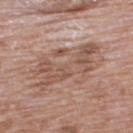| feature | finding |
|---|---|
| workup | total-body-photography surveillance lesion; no biopsy |
| anatomic site | the upper back |
| subject | male, in their 70s |
| tile lighting | white-light illumination |
| imaging modality | ~15 mm crop, total-body skin-cancer survey |